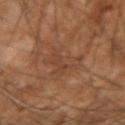Clinical impression:
Captured during whole-body skin photography for melanoma surveillance; the lesion was not biopsied.
Clinical summary:
Automated tile analysis of the lesion measured an area of roughly 9.5 mm², an outline eccentricity of about 0.5 (0 = round, 1 = elongated), and a shape-asymmetry score of about 0.55 (0 = symmetric). The software also gave a border-irregularity index near 7/10, internal color variation of about 3.5 on a 0–10 scale, and peripheral color asymmetry of about 1.5. Imaged with cross-polarized lighting. Cropped from a whole-body photographic skin survey; the tile spans about 15 mm. The recorded lesion diameter is about 4 mm. A male subject, approximately 55 years of age. From the right forearm.